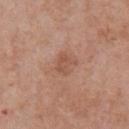Imaged with white-light lighting. A male patient, in their 70s. A 15 mm crop from a total-body photograph taken for skin-cancer surveillance. The lesion is on the chest. Measured at roughly 2.5 mm in maximum diameter.